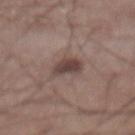follow-up: total-body-photography surveillance lesion; no biopsy
TBP lesion metrics: an average lesion color of about L≈42 a*≈16 b*≈19 (CIELAB), roughly 11 lightness units darker than nearby skin, and a normalized lesion–skin contrast near 9; a color-variation rating of about 2.5/10 and radial color variation of about 1
subject: male, roughly 55 years of age
acquisition: ~15 mm tile from a whole-body skin photo
lesion size: about 2.5 mm
location: the abdomen
illumination: white-light illumination